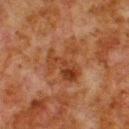Q: What is the imaging modality?
A: 15 mm crop, total-body photography
Q: Patient demographics?
A: male, aged approximately 80
Q: What is the anatomic site?
A: the upper back